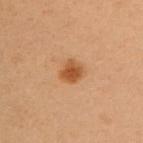Imaged during a routine full-body skin examination; the lesion was not biopsied and no histopathology is available.
This is a cross-polarized tile.
A female subject, approximately 50 years of age.
The lesion is on the chest.
A region of skin cropped from a whole-body photographic capture, roughly 15 mm wide.
The recorded lesion diameter is about 2.5 mm.